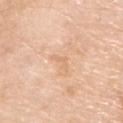Clinical impression: The lesion was photographed on a routine skin check and not biopsied; there is no pathology result. Acquisition and patient details: A male subject, aged approximately 80. About 3 mm across. A roughly 15 mm field-of-view crop from a total-body skin photograph. The lesion is on the back.